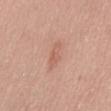  biopsy_status: not biopsied; imaged during a skin examination
  image:
    source: total-body photography crop
    field_of_view_mm: 15
  lesion_size:
    long_diameter_mm_approx: 3.5
  site: front of the torso
  automated_metrics:
    border_irregularity_0_10: 3.5
    peripheral_color_asymmetry: 0.5
    nevus_likeness_0_100: 15
    lesion_detection_confidence_0_100: 100
  patient:
    sex: male
    age_approx: 50